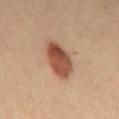Case summary:
– workup — no biopsy performed (imaged during a skin exam)
– body site — the back
– image-analysis metrics — an eccentricity of roughly 0.75 and a shape-asymmetry score of about 0.15 (0 = symmetric); about 12 CIELAB-L* units darker than the surrounding skin and a lesion-to-skin contrast of about 10.5 (normalized; higher = more distinct); a border-irregularity rating of about 1.5/10, a within-lesion color-variation index near 5/10, and a peripheral color-asymmetry measure near 2; a detector confidence of about 100 out of 100 that the crop contains a lesion
– image — ~15 mm tile from a whole-body skin photo
– lesion size — ≈4.5 mm
– subject — female, aged 28 to 32